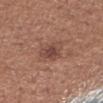<record>
<biopsy_status>not biopsied; imaged during a skin examination</biopsy_status>
<automated_metrics>
  <area_mm2_approx>6.5</area_mm2_approx>
  <eccentricity>0.55</eccentricity>
  <shape_asymmetry>0.35</shape_asymmetry>
  <cielab_L>45</cielab_L>
  <cielab_a>20</cielab_a>
  <cielab_b>25</cielab_b>
  <vs_skin_darker_L>8.0</vs_skin_darker_L>
  <vs_skin_contrast_norm>7.0</vs_skin_contrast_norm>
  <nevus_likeness_0_100>60</nevus_likeness_0_100>
  <lesion_detection_confidence_0_100>100</lesion_detection_confidence_0_100>
</automated_metrics>
<patient>
  <sex>female</sex>
  <age_approx>45</age_approx>
</patient>
<site>right forearm</site>
<lesion_size>
  <long_diameter_mm_approx>3.0</long_diameter_mm_approx>
</lesion_size>
<image>
  <source>total-body photography crop</source>
  <field_of_view_mm>15</field_of_view_mm>
</image>
<lighting>white-light</lighting>
</record>